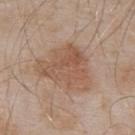Recorded during total-body skin imaging; not selected for excision or biopsy. The subject is a male about 55 years old. From the back. An algorithmic analysis of the crop reported an area of roughly 22 mm² and a shape eccentricity near 0.6. It also reported a lesion-to-skin contrast of about 6.5 (normalized; higher = more distinct). A lesion tile, about 15 mm wide, cut from a 3D total-body photograph.